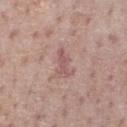{
  "biopsy_status": "not biopsied; imaged during a skin examination",
  "patient": {
    "sex": "male",
    "age_approx": 75
  },
  "lesion_size": {
    "long_diameter_mm_approx": 3.5
  },
  "site": "chest",
  "image": {
    "source": "total-body photography crop",
    "field_of_view_mm": 15
  },
  "automated_metrics": {
    "area_mm2_approx": 4.0,
    "eccentricity": 0.9,
    "shape_asymmetry": 0.35,
    "cielab_L": 55,
    "cielab_a": 22,
    "cielab_b": 21,
    "vs_skin_contrast_norm": 6.0,
    "border_irregularity_0_10": 4.5,
    "nevus_likeness_0_100": 0
  }
}